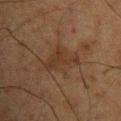Clinical impression: Captured during whole-body skin photography for melanoma surveillance; the lesion was not biopsied. Acquisition and patient details: A lesion tile, about 15 mm wide, cut from a 3D total-body photograph. This is a cross-polarized tile. The subject is a male roughly 60 years of age. Automated image analysis of the tile measured a mean CIELAB color near L≈27 a*≈14 b*≈24, a lesion–skin lightness drop of about 5, and a normalized border contrast of about 6. The analysis additionally found a border-irregularity rating of about 6/10, a color-variation rating of about 1.5/10, and radial color variation of about 0.5. The analysis additionally found an automated nevus-likeness rating near 0 out of 100 and a detector confidence of about 100 out of 100 that the crop contains a lesion. Longest diameter approximately 5 mm. From the upper back.